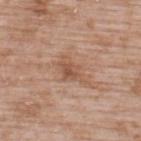The lesion was tiled from a total-body skin photograph and was not biopsied. A 15 mm close-up extracted from a 3D total-body photography capture. From the upper back. A male subject, approximately 50 years of age. The tile uses white-light illumination.